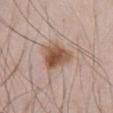follow-up = catalogued during a skin exam; not biopsied | anatomic site = the abdomen | lighting = white-light | acquisition = ~15 mm tile from a whole-body skin photo | subject = male, aged around 55 | size = ~4 mm (longest diameter) | TBP lesion metrics = an area of roughly 10 mm².